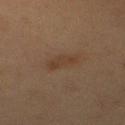{
  "biopsy_status": "not biopsied; imaged during a skin examination",
  "lighting": "cross-polarized",
  "patient": {
    "sex": "female",
    "age_approx": 40
  },
  "image": {
    "source": "total-body photography crop",
    "field_of_view_mm": 15
  },
  "site": "back",
  "lesion_size": {
    "long_diameter_mm_approx": 3.0
  }
}